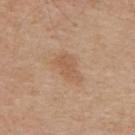Notes:
– subject · male, roughly 65 years of age
– body site · the upper back
– image · 15 mm crop, total-body photography
– illumination · white-light illumination